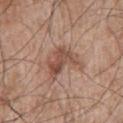Case summary:
- follow-up: imaged on a skin check; not biopsied
- body site: the arm
- illumination: white-light
- patient: male, roughly 65 years of age
- image: 15 mm crop, total-body photography